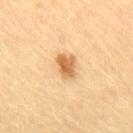A male patient approximately 85 years of age or older. A close-up tile cropped from a whole-body skin photograph, about 15 mm across. The lesion is on the lower back. An algorithmic analysis of the crop reported a mean CIELAB color near L≈56 a*≈19 b*≈38 and a normalized border contrast of about 8.5. The software also gave lesion-presence confidence of about 100/100. Approximately 3 mm at its widest. This is a cross-polarized tile.